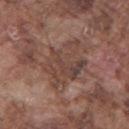| key | value |
|---|---|
| workup | total-body-photography surveillance lesion; no biopsy |